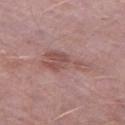notes: imaged on a skin check; not biopsied | patient: male, aged 48–52 | lesion diameter: ≈6 mm | imaging modality: ~15 mm crop, total-body skin-cancer survey | site: the left thigh | illumination: white-light.